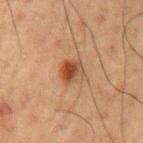Impression: The lesion was tiled from a total-body skin photograph and was not biopsied. Background: The recorded lesion diameter is about 3 mm. Located on the left upper arm. Cropped from a total-body skin-imaging series; the visible field is about 15 mm. A male subject, about 60 years old. This is a cross-polarized tile.